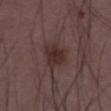This lesion was catalogued during total-body skin photography and was not selected for biopsy.
The lesion is on the left thigh.
Imaged with white-light lighting.
The lesion's longest dimension is about 3.5 mm.
A male subject, aged 48–52.
A region of skin cropped from a whole-body photographic capture, roughly 15 mm wide.